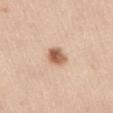Case summary:
• location · the front of the torso
• patient · female, aged 28 to 32
• acquisition · 15 mm crop, total-body photography
• tile lighting · white-light illumination